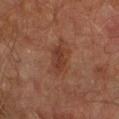The lesion was tiled from a total-body skin photograph and was not biopsied. The lesion is on the arm. A male patient roughly 75 years of age. This is a cross-polarized tile. A region of skin cropped from a whole-body photographic capture, roughly 15 mm wide.